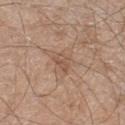Notes:
- follow-up — no biopsy performed (imaged during a skin exam)
- acquisition — total-body-photography crop, ~15 mm field of view
- patient — male, aged approximately 60
- lesion diameter — ~2.5 mm (longest diameter)
- illumination — white-light illumination
- location — the left lower leg